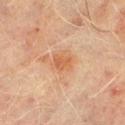This lesion was catalogued during total-body skin photography and was not selected for biopsy. The lesion is on the right lower leg. A lesion tile, about 15 mm wide, cut from a 3D total-body photograph. The patient is a male aged around 70. Automated tile analysis of the lesion measured a shape eccentricity near 0.7 and a symmetry-axis asymmetry near 0.15. It also reported a mean CIELAB color near L≈49 a*≈21 b*≈32, roughly 7 lightness units darker than nearby skin, and a normalized border contrast of about 6.5. The analysis additionally found a color-variation rating of about 2.5/10. Approximately 3 mm at its widest.